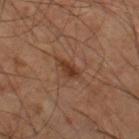Captured during whole-body skin photography for melanoma surveillance; the lesion was not biopsied.
A male subject aged around 60.
The lesion is located on the right thigh.
A close-up tile cropped from a whole-body skin photograph, about 15 mm across.
Imaged with cross-polarized lighting.